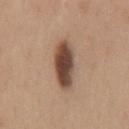Q: Is there a histopathology result?
A: total-body-photography surveillance lesion; no biopsy
Q: What kind of image is this?
A: 15 mm crop, total-body photography
Q: Where on the body is the lesion?
A: the mid back
Q: What are the patient's age and sex?
A: male, aged around 35